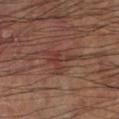Assessment:
Imaged during a routine full-body skin examination; the lesion was not biopsied and no histopathology is available.
Background:
Cropped from a total-body skin-imaging series; the visible field is about 15 mm. The patient is a male aged around 60. The lesion is on the left lower leg.